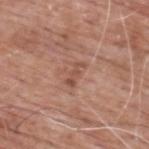• notes: imaged on a skin check; not biopsied
• size: ~3 mm (longest diameter)
• automated metrics: a lesion color around L≈51 a*≈22 b*≈28 in CIELAB and a lesion-to-skin contrast of about 6 (normalized; higher = more distinct); a border-irregularity index near 5.5/10, a within-lesion color-variation index near 0/10, and a peripheral color-asymmetry measure near 0
• location: the upper back
• lighting: white-light illumination
• patient: male, aged approximately 60
• image source: ~15 mm crop, total-body skin-cancer survey